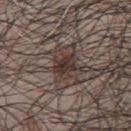Impression: This lesion was catalogued during total-body skin photography and was not selected for biopsy. Background: The lesion is on the chest. Longest diameter approximately 3 mm. A close-up tile cropped from a whole-body skin photograph, about 15 mm across. The tile uses white-light illumination. A male subject, approximately 50 years of age.